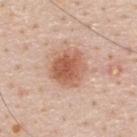Assessment: Captured during whole-body skin photography for melanoma surveillance; the lesion was not biopsied. Acquisition and patient details: The lesion is on the upper back. Automated image analysis of the tile measured a footprint of about 14 mm² and a shape-asymmetry score of about 0.15 (0 = symmetric). The software also gave an automated nevus-likeness rating near 100 out of 100 and lesion-presence confidence of about 100/100. The patient is a male in their 60s. The lesion's longest dimension is about 4.5 mm. The tile uses white-light illumination. A 15 mm close-up tile from a total-body photography series done for melanoma screening.